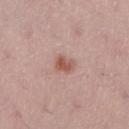This lesion was catalogued during total-body skin photography and was not selected for biopsy. The patient is a female roughly 50 years of age. A 15 mm crop from a total-body photograph taken for skin-cancer surveillance. From the right lower leg.